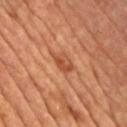Q: Is there a histopathology result?
A: imaged on a skin check; not biopsied
Q: Illumination type?
A: cross-polarized
Q: What is the lesion's diameter?
A: ~3 mm (longest diameter)
Q: What is the anatomic site?
A: the upper back
Q: Patient demographics?
A: male, in their mid-60s
Q: What is the imaging modality?
A: ~15 mm tile from a whole-body skin photo